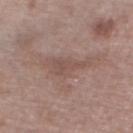Q: Lesion size?
A: ≈3.5 mm
Q: What are the patient's age and sex?
A: male, aged 63–67
Q: What lighting was used for the tile?
A: white-light illumination
Q: Where on the body is the lesion?
A: the left lower leg
Q: What is the imaging modality?
A: ~15 mm crop, total-body skin-cancer survey